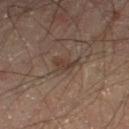patient: male, roughly 50 years of age | site: the right lower leg | diameter: ≈3 mm | image source: total-body-photography crop, ~15 mm field of view | lighting: cross-polarized | image-analysis metrics: a mean CIELAB color near L≈33 a*≈13 b*≈21, a lesion–skin lightness drop of about 6, and a lesion-to-skin contrast of about 6 (normalized; higher = more distinct); a border-irregularity index near 4/10 and a within-lesion color-variation index near 3/10.